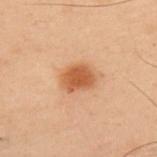Notes:
* biopsy status: catalogued during a skin exam; not biopsied
* patient: male, in their mid-50s
* illumination: cross-polarized illumination
* site: the upper back
* imaging modality: ~15 mm tile from a whole-body skin photo